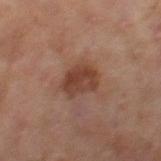Part of a total-body skin-imaging series; this lesion was reviewed on a skin check and was not flagged for biopsy. From the right thigh. The patient is a female aged approximately 60. A region of skin cropped from a whole-body photographic capture, roughly 15 mm wide. About 4 mm across.